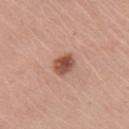The lesion was photographed on a routine skin check and not biopsied; there is no pathology result. Imaged with white-light lighting. A 15 mm close-up extracted from a 3D total-body photography capture. Located on the front of the torso. A female subject, roughly 65 years of age.